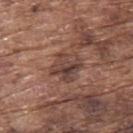workup: total-body-photography surveillance lesion; no biopsy | diameter: ≈3.5 mm | image-analysis metrics: a footprint of about 10 mm² and an eccentricity of roughly 0.45; a mean CIELAB color near L≈42 a*≈19 b*≈23 and a lesion-to-skin contrast of about 7.5 (normalized; higher = more distinct); an automated nevus-likeness rating near 0 out of 100 and a lesion-detection confidence of about 75/100 | site: the upper back | patient: male, about 75 years old | image source: 15 mm crop, total-body photography | tile lighting: white-light.